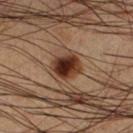Notes:
* biopsy status · no biopsy performed (imaged during a skin exam)
* subject · male, about 65 years old
* location · the left lower leg
* image-analysis metrics · an area of roughly 10 mm² and a symmetry-axis asymmetry near 0.15
* image · total-body-photography crop, ~15 mm field of view
* illumination · cross-polarized